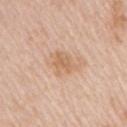Impression:
No biopsy was performed on this lesion — it was imaged during a full skin examination and was not determined to be concerning.
Acquisition and patient details:
The patient is a female in their mid- to late 40s. Measured at roughly 3.5 mm in maximum diameter. Automated image analysis of the tile measured a lesion area of about 6.5 mm², an eccentricity of roughly 0.75, and a symmetry-axis asymmetry near 0.35. The analysis additionally found a lesion color around L≈64 a*≈19 b*≈34 in CIELAB, a lesion–skin lightness drop of about 8, and a normalized border contrast of about 6. The software also gave a classifier nevus-likeness of about 15/100 and lesion-presence confidence of about 100/100. On the right upper arm. Cropped from a total-body skin-imaging series; the visible field is about 15 mm.